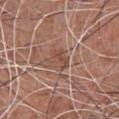lesion diameter: ≈3 mm | subject: male, about 55 years old | lighting: white-light | image-analysis metrics: a within-lesion color-variation index near 4/10; a classifier nevus-likeness of about 0/100 | image: 15 mm crop, total-body photography | anatomic site: the chest.